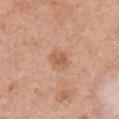Clinical impression: Captured during whole-body skin photography for melanoma surveillance; the lesion was not biopsied. Context: The lesion is on the chest. Captured under white-light illumination. Automated tile analysis of the lesion measured border irregularity of about 2 on a 0–10 scale, a color-variation rating of about 2/10, and a peripheral color-asymmetry measure near 0.5. It also reported a classifier nevus-likeness of about 20/100. Cropped from a whole-body photographic skin survey; the tile spans about 15 mm. The subject is a female aged 58 to 62. The lesion's longest dimension is about 2.5 mm.